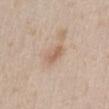Recorded during total-body skin imaging; not selected for excision or biopsy. From the abdomen. A female patient about 30 years old. Automated image analysis of the tile measured an area of roughly 4 mm² and a shape eccentricity near 0.8. And it measured a mean CIELAB color near L≈61 a*≈17 b*≈30, about 9 CIELAB-L* units darker than the surrounding skin, and a lesion-to-skin contrast of about 6 (normalized; higher = more distinct). It also reported a border-irregularity index near 2/10 and peripheral color asymmetry of about 0.5. The software also gave an automated nevus-likeness rating near 20 out of 100 and a detector confidence of about 100 out of 100 that the crop contains a lesion. This image is a 15 mm lesion crop taken from a total-body photograph. This is a white-light tile.